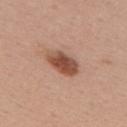Part of a total-body skin-imaging series; this lesion was reviewed on a skin check and was not flagged for biopsy. From the mid back. About 4.5 mm across. The tile uses white-light illumination. A region of skin cropped from a whole-body photographic capture, roughly 15 mm wide. A female subject, about 55 years old.